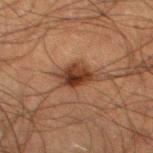Case summary:
- follow-up · no biopsy performed (imaged during a skin exam)
- anatomic site · the left thigh
- patient · male, about 50 years old
- image source · 15 mm crop, total-body photography